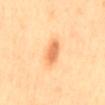The lesion was photographed on a routine skin check and not biopsied; there is no pathology result.
Imaged with cross-polarized lighting.
A female subject, in their mid- to late 40s.
Measured at roughly 4 mm in maximum diameter.
Cropped from a whole-body photographic skin survey; the tile spans about 15 mm.
Located on the mid back.